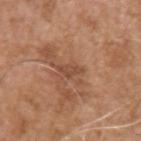Clinical impression: Recorded during total-body skin imaging; not selected for excision or biopsy. Context: About 3 mm across. Located on the left upper arm. This image is a 15 mm lesion crop taken from a total-body photograph. This is a white-light tile. The lesion-visualizer software estimated a lesion-detection confidence of about 100/100. The subject is a male aged around 75.